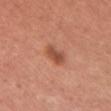biopsy_status: not biopsied; imaged during a skin examination
site: upper back
image:
  source: total-body photography crop
  field_of_view_mm: 15
patient:
  sex: female
  age_approx: 35
lesion_size:
  long_diameter_mm_approx: 3.0